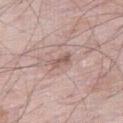Impression: Part of a total-body skin-imaging series; this lesion was reviewed on a skin check and was not flagged for biopsy. Acquisition and patient details: This is a white-light tile. Cropped from a whole-body photographic skin survey; the tile spans about 15 mm. The recorded lesion diameter is about 2.5 mm. The lesion is on the right thigh. An algorithmic analysis of the crop reported a mean CIELAB color near L≈57 a*≈18 b*≈23, roughly 9 lightness units darker than nearby skin, and a normalized lesion–skin contrast near 6.5. It also reported a nevus-likeness score of about 0/100. A male subject about 65 years old.